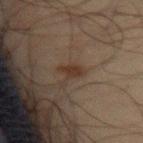No biopsy was performed on this lesion — it was imaged during a full skin examination and was not determined to be concerning.
Located on the front of the torso.
This is a cross-polarized tile.
A 15 mm close-up tile from a total-body photography series done for melanoma screening.
A male subject, aged 68–72.